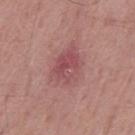biopsy status: no biopsy performed (imaged during a skin exam).